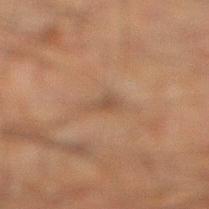Clinical impression:
This lesion was catalogued during total-body skin photography and was not selected for biopsy.
Background:
Captured under cross-polarized illumination. A lesion tile, about 15 mm wide, cut from a 3D total-body photograph. The lesion is on the right lower leg. The subject is a male aged around 50. Measured at roughly 2.5 mm in maximum diameter.